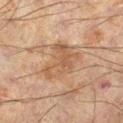The lesion was photographed on a routine skin check and not biopsied; there is no pathology result. A region of skin cropped from a whole-body photographic capture, roughly 15 mm wide. The lesion is on the left leg. A male subject, aged around 60.